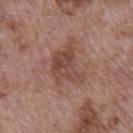Q: What kind of image is this?
A: total-body-photography crop, ~15 mm field of view
Q: What are the patient's age and sex?
A: male, aged 68–72
Q: Lesion location?
A: the mid back